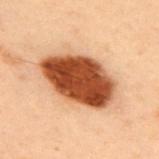{"image": {"source": "total-body photography crop", "field_of_view_mm": 15}, "patient": {"sex": "male", "age_approx": 55}, "lighting": "cross-polarized", "site": "upper back", "automated_metrics": {"eccentricity": 0.8, "shape_asymmetry": 0.1, "nevus_likeness_0_100": 100, "lesion_detection_confidence_0_100": 100}}